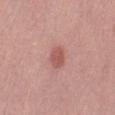| key | value |
|---|---|
| tile lighting | white-light illumination |
| subject | female, aged around 40 |
| lesion diameter | ~2.5 mm (longest diameter) |
| body site | the back |
| image-analysis metrics | a lesion–skin lightness drop of about 10; a border-irregularity rating of about 1/10, a within-lesion color-variation index near 2/10, and peripheral color asymmetry of about 0.5; an automated nevus-likeness rating near 90 out of 100 and a lesion-detection confidence of about 100/100 |
| image | 15 mm crop, total-body photography |